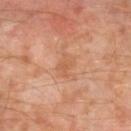biopsy status: no biopsy performed (imaged during a skin exam) | patient: male, aged 58–62 | lesion size: ≈3 mm | body site: the right lower leg | tile lighting: cross-polarized illumination | imaging modality: ~15 mm crop, total-body skin-cancer survey.